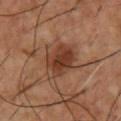The lesion was tiled from a total-body skin photograph and was not biopsied. Located on the chest. The lesion-visualizer software estimated a lesion area of about 14 mm², a shape eccentricity near 0.7, and two-axis asymmetry of about 0.2. A roughly 15 mm field-of-view crop from a total-body skin photograph. A male patient roughly 55 years of age.